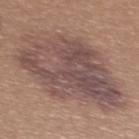A lesion tile, about 15 mm wide, cut from a 3D total-body photograph. Longest diameter approximately 11 mm. Captured under white-light illumination. A female subject aged 38 to 42. The lesion is on the upper back. On biopsy, histopathology showed a dysplastic (Clark) nevus.